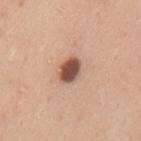On the mid back.
A male subject, about 35 years old.
A close-up tile cropped from a whole-body skin photograph, about 15 mm across.
Captured under white-light illumination.
About 3.5 mm across.